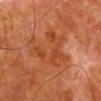Notes:
- location · the left lower leg
- lighting · cross-polarized
- subject · male, roughly 80 years of age
- image source · ~15 mm crop, total-body skin-cancer survey
- size · about 6.5 mm